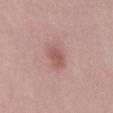<record>
<patient>
  <sex>male</sex>
  <age_approx>30</age_approx>
</patient>
<automated_metrics>
  <area_mm2_approx>4.5</area_mm2_approx>
  <eccentricity>0.8</eccentricity>
  <shape_asymmetry>0.2</shape_asymmetry>
  <nevus_likeness_0_100>60</nevus_likeness_0_100>
</automated_metrics>
<lighting>white-light</lighting>
<lesion_size>
  <long_diameter_mm_approx>3.0</long_diameter_mm_approx>
</lesion_size>
<image>
  <source>total-body photography crop</source>
  <field_of_view_mm>15</field_of_view_mm>
</image>
</record>